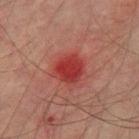follow-up=catalogued during a skin exam; not biopsied
site=the right thigh
subject=male, about 75 years old
acquisition=~15 mm tile from a whole-body skin photo
diameter=≈3.5 mm